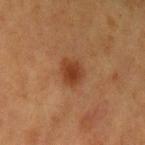Clinical impression:
This lesion was catalogued during total-body skin photography and was not selected for biopsy.
Acquisition and patient details:
The tile uses cross-polarized illumination. Measured at roughly 3 mm in maximum diameter. On the left upper arm. A female patient, in their mid- to late 50s. The total-body-photography lesion software estimated a lesion-to-skin contrast of about 8 (normalized; higher = more distinct). A region of skin cropped from a whole-body photographic capture, roughly 15 mm wide.